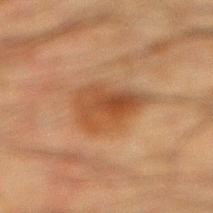<tbp_lesion>
  <biopsy_status>not biopsied; imaged during a skin examination</biopsy_status>
  <automated_metrics>
    <cielab_L>37</cielab_L>
    <cielab_a>19</cielab_a>
    <cielab_b>30</cielab_b>
    <vs_skin_darker_L>9.0</vs_skin_darker_L>
    <vs_skin_contrast_norm>8.0</vs_skin_contrast_norm>
    <nevus_likeness_0_100>55</nevus_likeness_0_100>
    <lesion_detection_confidence_0_100>100</lesion_detection_confidence_0_100>
  </automated_metrics>
  <site>right lower leg</site>
  <image>
    <source>total-body photography crop</source>
    <field_of_view_mm>15</field_of_view_mm>
  </image>
  <lighting>cross-polarized</lighting>
  <patient>
    <sex>male</sex>
    <age_approx>35</age_approx>
  </patient>
  <lesion_size>
    <long_diameter_mm_approx>5.0</long_diameter_mm_approx>
  </lesion_size>
</tbp_lesion>